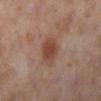<lesion>
<biopsy_status>not biopsied; imaged during a skin examination</biopsy_status>
<image>
  <source>total-body photography crop</source>
  <field_of_view_mm>15</field_of_view_mm>
</image>
<site>left lower leg</site>
<lighting>cross-polarized</lighting>
<patient>
  <sex>female</sex>
  <age_approx>40</age_approx>
</patient>
<lesion_size>
  <long_diameter_mm_approx>3.5</long_diameter_mm_approx>
</lesion_size>
</lesion>